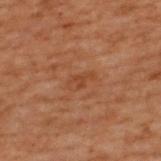Background:
On the upper back. A male patient aged around 70. Imaged with cross-polarized lighting. This image is a 15 mm lesion crop taken from a total-body photograph.